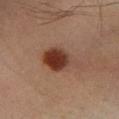This lesion was catalogued during total-body skin photography and was not selected for biopsy. A male patient, aged approximately 40. About 5 mm across. Automated image analysis of the tile measured an area of roughly 12 mm², an eccentricity of roughly 0.75, and a symmetry-axis asymmetry near 0.25. It also reported a border-irregularity rating of about 3/10 and internal color variation of about 7 on a 0–10 scale. The lesion is located on the leg. Captured under cross-polarized illumination. A close-up tile cropped from a whole-body skin photograph, about 15 mm across.